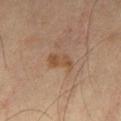• workup · total-body-photography surveillance lesion; no biopsy
• image source · ~15 mm tile from a whole-body skin photo
• patient · male, aged around 65
• lighting · cross-polarized
• anatomic site · the left thigh